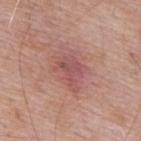<tbp_lesion>
<biopsy_status>not biopsied; imaged during a skin examination</biopsy_status>
<site>back</site>
<lighting>white-light</lighting>
<lesion_size>
  <long_diameter_mm_approx>4.0</long_diameter_mm_approx>
</lesion_size>
<patient>
  <sex>male</sex>
  <age_approx>60</age_approx>
</patient>
<image>
  <source>total-body photography crop</source>
  <field_of_view_mm>15</field_of_view_mm>
</image>
</tbp_lesion>